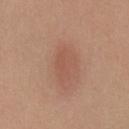follow-up: catalogued during a skin exam; not biopsied | subject: male, in their 30s | lesion size: ≈4 mm | automated lesion analysis: a lesion area of about 7 mm², an outline eccentricity of about 0.85 (0 = round, 1 = elongated), and a shape-asymmetry score of about 0.3 (0 = symmetric); a lesion-detection confidence of about 100/100 | illumination: white-light illumination | acquisition: 15 mm crop, total-body photography | anatomic site: the chest.